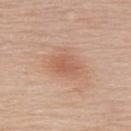Findings:
- biopsy status: no biopsy performed (imaged during a skin exam)
- site: the back
- image source: ~15 mm crop, total-body skin-cancer survey
- subject: female, about 65 years old
- lesion diameter: ~3.5 mm (longest diameter)
- TBP lesion metrics: a lesion area of about 7 mm², an outline eccentricity of about 0.65 (0 = round, 1 = elongated), and two-axis asymmetry of about 0.2
- illumination: white-light illumination